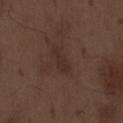Q: Is there a histopathology result?
A: no biopsy performed (imaged during a skin exam)
Q: What are the patient's age and sex?
A: male, aged around 70
Q: How was this image acquired?
A: ~15 mm tile from a whole-body skin photo
Q: What did automated image analysis measure?
A: about 5 CIELAB-L* units darker than the surrounding skin and a lesion-to-skin contrast of about 5.5 (normalized; higher = more distinct); a border-irregularity rating of about 4/10, a color-variation rating of about 1/10, and a peripheral color-asymmetry measure near 0.5; lesion-presence confidence of about 100/100
Q: Lesion location?
A: the abdomen
Q: Lesion size?
A: ~3.5 mm (longest diameter)
Q: How was the tile lit?
A: white-light illumination